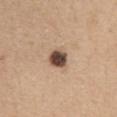Part of a total-body skin-imaging series; this lesion was reviewed on a skin check and was not flagged for biopsy. The patient is a female in their mid- to late 40s. Cropped from a whole-body photographic skin survey; the tile spans about 15 mm. From the abdomen.